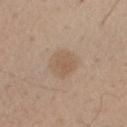Assessment: The lesion was tiled from a total-body skin photograph and was not biopsied. Context: Automated tile analysis of the lesion measured a border-irregularity rating of about 1.5/10, internal color variation of about 2 on a 0–10 scale, and radial color variation of about 0.5. Captured under white-light illumination. Located on the right upper arm. Cropped from a total-body skin-imaging series; the visible field is about 15 mm. Longest diameter approximately 3.5 mm. The subject is a male aged around 55.